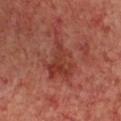Notes:
• follow-up · imaged on a skin check; not biopsied
• illumination · cross-polarized illumination
• location · the chest
• automated metrics · a mean CIELAB color near L≈38 a*≈29 b*≈28 and a lesion–skin lightness drop of about 7; a border-irregularity index near 7.5/10, a color-variation rating of about 4/10, and a peripheral color-asymmetry measure near 1.5; a nevus-likeness score of about 0/100 and a lesion-detection confidence of about 100/100
• acquisition · ~15 mm crop, total-body skin-cancer survey
• subject · female, in their mid-40s
• size · ≈6 mm